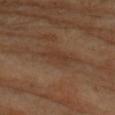<lesion>
<biopsy_status>not biopsied; imaged during a skin examination</biopsy_status>
<lighting>cross-polarized</lighting>
<lesion_size>
  <long_diameter_mm_approx>4.0</long_diameter_mm_approx>
</lesion_size>
<site>arm</site>
<image>
  <source>total-body photography crop</source>
  <field_of_view_mm>15</field_of_view_mm>
</image>
<patient>
  <sex>female</sex>
  <age_approx>70</age_approx>
</patient>
<automated_metrics>
  <area_mm2_approx>4.5</area_mm2_approx>
  <eccentricity>0.95</eccentricity>
  <shape_asymmetry>0.35</shape_asymmetry>
  <cielab_L>37</cielab_L>
  <cielab_a>18</cielab_a>
  <cielab_b>28</cielab_b>
  <vs_skin_darker_L>6.0</vs_skin_darker_L>
  <vs_skin_contrast_norm>6.0</vs_skin_contrast_norm>
  <color_variation_0_10>1.0</color_variation_0_10>
  <peripheral_color_asymmetry>0.0</peripheral_color_asymmetry>
</automated_metrics>
</lesion>